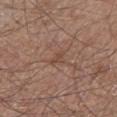This lesion was catalogued during total-body skin photography and was not selected for biopsy. From the left lower leg. A region of skin cropped from a whole-body photographic capture, roughly 15 mm wide. Longest diameter approximately 2.5 mm. Automated tile analysis of the lesion measured a border-irregularity index near 4.5/10, a within-lesion color-variation index near 0.5/10, and a peripheral color-asymmetry measure near 0. A male patient aged 58 to 62.